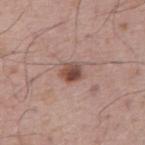Context:
A male patient, aged around 65. The lesion is on the abdomen. A roughly 15 mm field-of-view crop from a total-body skin photograph. An algorithmic analysis of the crop reported a mean CIELAB color near L≈49 a*≈20 b*≈24, about 13 CIELAB-L* units darker than the surrounding skin, and a lesion-to-skin contrast of about 9 (normalized; higher = more distinct). The software also gave a classifier nevus-likeness of about 95/100 and lesion-presence confidence of about 100/100. This is a white-light tile. Longest diameter approximately 3 mm.